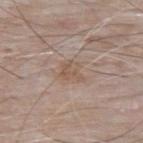Findings:
– acquisition: total-body-photography crop, ~15 mm field of view
– patient: male, roughly 65 years of age
– lighting: white-light illumination
– location: the upper back
– lesion diameter: ≈3 mm